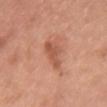follow-up: catalogued during a skin exam; not biopsied
location: the chest
lighting: white-light illumination
image: ~15 mm crop, total-body skin-cancer survey
subject: female, approximately 60 years of age